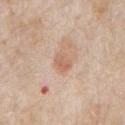Acquisition and patient details:
On the chest. Approximately 2.5 mm at its widest. The tile uses white-light illumination. A male patient aged 63–67. Automated image analysis of the tile measured a lesion area of about 4.5 mm² and an eccentricity of roughly 0.6. The software also gave a lesion color around L≈63 a*≈20 b*≈31 in CIELAB, a lesion–skin lightness drop of about 8, and a lesion-to-skin contrast of about 5.5 (normalized; higher = more distinct). And it measured a border-irregularity rating of about 1.5/10, a within-lesion color-variation index near 3/10, and radial color variation of about 1. A 15 mm close-up tile from a total-body photography series done for melanoma screening.